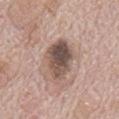follow-up: imaged on a skin check; not biopsied
patient: male, aged 68–72
tile lighting: white-light
site: the mid back
imaging modality: ~15 mm crop, total-body skin-cancer survey
automated metrics: a classifier nevus-likeness of about 75/100 and lesion-presence confidence of about 100/100